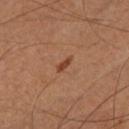biopsy_status: not biopsied; imaged during a skin examination
automated_metrics:
  border_irregularity_0_10: 3.0
  color_variation_0_10: 0.0
  peripheral_color_asymmetry: 0.0
patient:
  sex: male
  age_approx: 60
lesion_size:
  long_diameter_mm_approx: 2.5
lighting: cross-polarized
site: left lower leg
image:
  source: total-body photography crop
  field_of_view_mm: 15